Located on the arm; a lesion tile, about 15 mm wide, cut from a 3D total-body photograph; the patient is a male approximately 45 years of age: 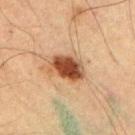TBP lesion metrics=a lesion color around L≈43 a*≈22 b*≈32 in CIELAB and a normalized border contrast of about 12.5 | illumination=cross-polarized | size=~4 mm (longest diameter) | diagnosis=a dysplastic (Clark) nevus.